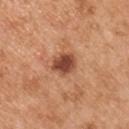Impression: Part of a total-body skin-imaging series; this lesion was reviewed on a skin check and was not flagged for biopsy. Context: A male subject aged approximately 55. This is a white-light tile. Cropped from a whole-body photographic skin survey; the tile spans about 15 mm. Approximately 3 mm at its widest. The lesion is located on the right upper arm.